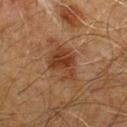<lesion>
  <biopsy_status>not biopsied; imaged during a skin examination</biopsy_status>
  <patient>
    <sex>male</sex>
    <age_approx>60</age_approx>
  </patient>
  <automated_metrics>
    <area_mm2_approx>10.0</area_mm2_approx>
    <shape_asymmetry>0.35</shape_asymmetry>
  </automated_metrics>
  <site>chest</site>
  <lesion_size>
    <long_diameter_mm_approx>4.5</long_diameter_mm_approx>
  </lesion_size>
  <image>
    <source>total-body photography crop</source>
    <field_of_view_mm>15</field_of_view_mm>
  </image>
</lesion>